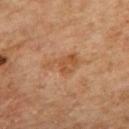<tbp_lesion>
<biopsy_status>not biopsied; imaged during a skin examination</biopsy_status>
<lighting>cross-polarized</lighting>
<patient>
  <sex>female</sex>
  <age_approx>60</age_approx>
</patient>
<site>upper back</site>
<automated_metrics>
  <cielab_L>48</cielab_L>
  <cielab_a>21</cielab_a>
  <cielab_b>34</cielab_b>
  <vs_skin_contrast_norm>6.0</vs_skin_contrast_norm>
</automated_metrics>
<image>
  <source>total-body photography crop</source>
  <field_of_view_mm>15</field_of_view_mm>
</image>
</tbp_lesion>